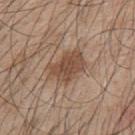<lesion>
  <biopsy_status>not biopsied; imaged during a skin examination</biopsy_status>
</lesion>